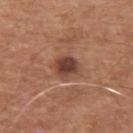Q: What is the anatomic site?
A: the left upper arm
Q: What are the patient's age and sex?
A: male, about 65 years old
Q: What kind of image is this?
A: 15 mm crop, total-body photography